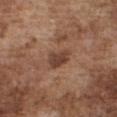workup — no biopsy performed (imaged during a skin exam)
lighting — white-light illumination
body site — the chest
subject — male, roughly 75 years of age
imaging modality — ~15 mm tile from a whole-body skin photo
automated metrics — a lesion area of about 5.5 mm² and two-axis asymmetry of about 0.3; an average lesion color of about L≈42 a*≈20 b*≈27 (CIELAB) and a lesion-to-skin contrast of about 8.5 (normalized; higher = more distinct); an automated nevus-likeness rating near 0 out of 100